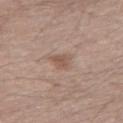Q: Was this lesion biopsied?
A: catalogued during a skin exam; not biopsied
Q: What did automated image analysis measure?
A: border irregularity of about 3.5 on a 0–10 scale and a within-lesion color-variation index near 1.5/10; a classifier nevus-likeness of about 10/100 and a detector confidence of about 100 out of 100 that the crop contains a lesion
Q: Illumination type?
A: white-light
Q: Who is the patient?
A: male, in their 30s
Q: What kind of image is this?
A: ~15 mm crop, total-body skin-cancer survey
Q: How large is the lesion?
A: ≈2.5 mm